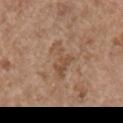workup: catalogued during a skin exam; not biopsied | patient: female, aged approximately 65 | size: ≈5 mm | location: the right upper arm | imaging modality: 15 mm crop, total-body photography.